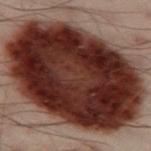{"biopsy_status": "not biopsied; imaged during a skin examination", "lesion_size": {"long_diameter_mm_approx": 14.5}, "image": {"source": "total-body photography crop", "field_of_view_mm": 15}, "lighting": "cross-polarized", "site": "left thigh", "automated_metrics": {"nevus_likeness_0_100": 80}, "patient": {"sex": "male", "age_approx": 50}}